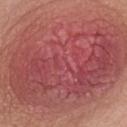Context:
A close-up tile cropped from a whole-body skin photograph, about 15 mm across. On the chest. A female patient in their mid-40s.